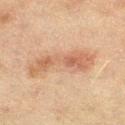Clinical summary:
The lesion is on the leg. A roughly 15 mm field-of-view crop from a total-body skin photograph. The patient is a female in their mid- to late 50s.